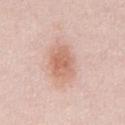workup — imaged on a skin check; not biopsied
location — the chest
lesion size — about 4 mm
illumination — white-light
subject — male, roughly 25 years of age
acquisition — 15 mm crop, total-body photography